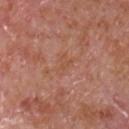Impression:
Part of a total-body skin-imaging series; this lesion was reviewed on a skin check and was not flagged for biopsy.
Background:
A 15 mm close-up extracted from a 3D total-body photography capture. The lesion is located on the front of the torso. An algorithmic analysis of the crop reported an area of roughly 3 mm², a shape eccentricity near 0.8, and two-axis asymmetry of about 0.35. And it measured an average lesion color of about L≈52 a*≈24 b*≈32 (CIELAB), about 4 CIELAB-L* units darker than the surrounding skin, and a lesion-to-skin contrast of about 4.5 (normalized; higher = more distinct). The software also gave a peripheral color-asymmetry measure near 0. And it measured a classifier nevus-likeness of about 0/100 and lesion-presence confidence of about 100/100. The recorded lesion diameter is about 2.5 mm. A male patient, about 65 years old.